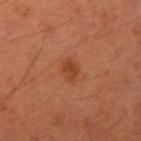location: the right upper arm
illumination: cross-polarized
subject: male, about 35 years old
image source: total-body-photography crop, ~15 mm field of view
automated lesion analysis: a lesion area of about 3.5 mm², a shape eccentricity near 0.6, and a shape-asymmetry score of about 0.2 (0 = symmetric); a lesion color around L≈41 a*≈27 b*≈34 in CIELAB and about 8 CIELAB-L* units darker than the surrounding skin; border irregularity of about 2 on a 0–10 scale, a color-variation rating of about 2/10, and radial color variation of about 1; a detector confidence of about 100 out of 100 that the crop contains a lesion
lesion size: ≈2.5 mm